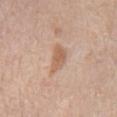Notes:
• workup · total-body-photography surveillance lesion; no biopsy
• body site · the chest
• patient · male, about 80 years old
• acquisition · ~15 mm tile from a whole-body skin photo
• lighting · white-light illumination
• diameter · ≈4 mm
• image-analysis metrics · a nevus-likeness score of about 25/100